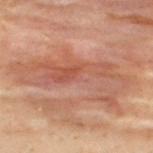Clinical impression: Recorded during total-body skin imaging; not selected for excision or biopsy. Acquisition and patient details: Imaged with cross-polarized lighting. Located on the upper back. The lesion's longest dimension is about 13.5 mm. A 15 mm close-up extracted from a 3D total-body photography capture. A male subject in their 30s.